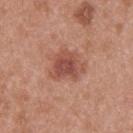notes: catalogued during a skin exam; not biopsied
acquisition: ~15 mm tile from a whole-body skin photo
tile lighting: white-light
patient: male, aged approximately 30
automated lesion analysis: a border-irregularity index near 4/10, a color-variation rating of about 3.5/10, and radial color variation of about 1; a classifier nevus-likeness of about 70/100 and lesion-presence confidence of about 100/100
diameter: ~4 mm (longest diameter)
anatomic site: the upper back